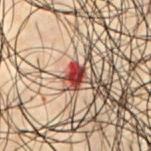Recorded during total-body skin imaging; not selected for excision or biopsy.
The lesion is located on the chest.
A male patient aged approximately 50.
Cropped from a total-body skin-imaging series; the visible field is about 15 mm.